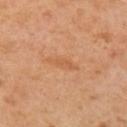• notes: imaged on a skin check; not biopsied
• subject: female, roughly 40 years of age
• anatomic site: the left upper arm
• image: total-body-photography crop, ~15 mm field of view
• lighting: cross-polarized